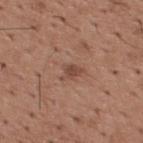Q: Was this lesion biopsied?
A: no biopsy performed (imaged during a skin exam)
Q: What are the patient's age and sex?
A: male, about 65 years old
Q: What is the imaging modality?
A: 15 mm crop, total-body photography
Q: How large is the lesion?
A: about 2.5 mm
Q: How was the tile lit?
A: white-light illumination
Q: What is the anatomic site?
A: the upper back
Q: Automated lesion metrics?
A: an area of roughly 3.5 mm², an eccentricity of roughly 0.75, and a shape-asymmetry score of about 0.45 (0 = symmetric); roughly 9 lightness units darker than nearby skin and a normalized lesion–skin contrast near 6.5; border irregularity of about 4.5 on a 0–10 scale, internal color variation of about 1.5 on a 0–10 scale, and a peripheral color-asymmetry measure near 0.5; a nevus-likeness score of about 35/100